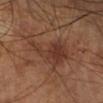Q: Was this lesion biopsied?
A: total-body-photography surveillance lesion; no biopsy
Q: What lighting was used for the tile?
A: cross-polarized illumination
Q: Where on the body is the lesion?
A: the right lower leg
Q: Patient demographics?
A: male, in their 70s
Q: Automated lesion metrics?
A: border irregularity of about 8 on a 0–10 scale, a within-lesion color-variation index near 4/10, and radial color variation of about 1; a nevus-likeness score of about 20/100 and lesion-presence confidence of about 100/100
Q: What kind of image is this?
A: ~15 mm tile from a whole-body skin photo